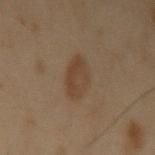The lesion was tiled from a total-body skin photograph and was not biopsied. A region of skin cropped from a whole-body photographic capture, roughly 15 mm wide. A male subject in their mid-50s. Captured under cross-polarized illumination. On the left upper arm. Approximately 3.5 mm at its widest.